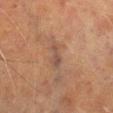{
  "biopsy_status": "not biopsied; imaged during a skin examination",
  "patient": {
    "sex": "male",
    "age_approx": 70
  },
  "site": "left lower leg",
  "lighting": "cross-polarized",
  "image": {
    "source": "total-body photography crop",
    "field_of_view_mm": 15
  },
  "lesion_size": {
    "long_diameter_mm_approx": 2.5
  }
}